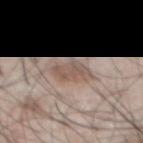notes: catalogued during a skin exam; not biopsied | image source: 15 mm crop, total-body photography | lesion diameter: ≈4 mm | patient: male, aged around 45 | automated lesion analysis: border irregularity of about 3 on a 0–10 scale, a color-variation rating of about 3/10, and a peripheral color-asymmetry measure near 1; an automated nevus-likeness rating near 95 out of 100 and a detector confidence of about 90 out of 100 that the crop contains a lesion | lighting: white-light | location: the front of the torso.